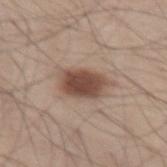This lesion was catalogued during total-body skin photography and was not selected for biopsy. A male subject, approximately 60 years of age. A 15 mm close-up tile from a total-body photography series done for melanoma screening. Located on the leg.